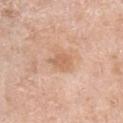{
  "biopsy_status": "not biopsied; imaged during a skin examination"
}